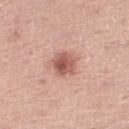{
  "image": {
    "source": "total-body photography crop",
    "field_of_view_mm": 15
  },
  "patient": {
    "sex": "male",
    "age_approx": 65
  },
  "site": "right lower leg",
  "lighting": "white-light",
  "automated_metrics": {
    "area_mm2_approx": 7.5,
    "eccentricity": 0.3,
    "shape_asymmetry": 0.2
  }
}